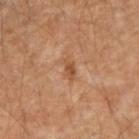workup: total-body-photography surveillance lesion; no biopsy
patient: male, aged around 55
acquisition: ~15 mm tile from a whole-body skin photo
location: the right forearm